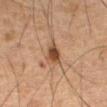<record>
  <biopsy_status>not biopsied; imaged during a skin examination</biopsy_status>
  <site>abdomen</site>
  <patient>
    <sex>male</sex>
    <age_approx>75</age_approx>
  </patient>
  <lesion_size>
    <long_diameter_mm_approx>2.5</long_diameter_mm_approx>
  </lesion_size>
  <lighting>cross-polarized</lighting>
  <image>
    <source>total-body photography crop</source>
    <field_of_view_mm>15</field_of_view_mm>
  </image>
</record>